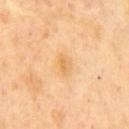notes=catalogued during a skin exam; not biopsied | imaging modality=total-body-photography crop, ~15 mm field of view | diameter=about 2.5 mm | lighting=cross-polarized illumination | body site=the front of the torso | automated metrics=a footprint of about 4 mm² and a shape eccentricity near 0.8; roughly 7 lightness units darker than nearby skin; border irregularity of about 2 on a 0–10 scale, a color-variation rating of about 2.5/10, and radial color variation of about 1 | patient=male, aged 48 to 52.